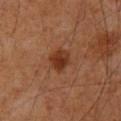Notes:
* workup — no biopsy performed (imaged during a skin exam)
* image — 15 mm crop, total-body photography
* automated metrics — an area of roughly 5.5 mm², a shape eccentricity near 0.5, and a symmetry-axis asymmetry near 0.2; a lesion color around L≈31 a*≈22 b*≈30 in CIELAB, about 9 CIELAB-L* units darker than the surrounding skin, and a normalized border contrast of about 9; a border-irregularity rating of about 2/10 and a within-lesion color-variation index near 3.5/10; lesion-presence confidence of about 100/100
* diameter — ~3 mm (longest diameter)
* tile lighting — cross-polarized
* body site — the back
* subject — male, aged approximately 60